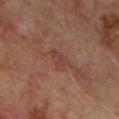A 15 mm close-up extracted from a 3D total-body photography capture. The lesion is located on the front of the torso. A male patient, in their mid- to late 60s.